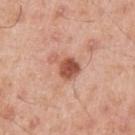image:
  source: total-body photography crop
  field_of_view_mm: 15
patient:
  sex: male
  age_approx: 55
lighting: white-light
lesion_size:
  long_diameter_mm_approx: 3.5
site: left upper arm